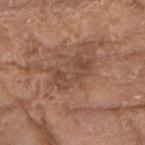biopsy_status: not biopsied; imaged during a skin examination
site: left forearm
lesion_size:
  long_diameter_mm_approx: 5.0
automated_metrics:
  nevus_likeness_0_100: 0
  lesion_detection_confidence_0_100: 95
image:
  source: total-body photography crop
  field_of_view_mm: 15
patient:
  sex: female
  age_approx: 75